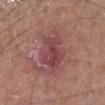Findings:
– automated lesion analysis · an average lesion color of about L≈44 a*≈25 b*≈19 (CIELAB), a lesion–skin lightness drop of about 9, and a normalized lesion–skin contrast near 7
– imaging modality · total-body-photography crop, ~15 mm field of view
– subject · male, in their mid- to late 50s
– anatomic site · the leg